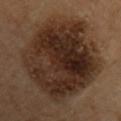Clinical impression: The lesion was photographed on a routine skin check and not biopsied; there is no pathology result. Acquisition and patient details: The lesion is located on the back. The tile uses cross-polarized illumination. About 11 mm across. Cropped from a total-body skin-imaging series; the visible field is about 15 mm. An algorithmic analysis of the crop reported a footprint of about 70 mm², an eccentricity of roughly 0.65, and two-axis asymmetry of about 0.2. The analysis additionally found border irregularity of about 2.5 on a 0–10 scale, a color-variation rating of about 7.5/10, and peripheral color asymmetry of about 3.